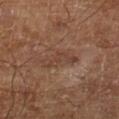Q: Was this lesion biopsied?
A: no biopsy performed (imaged during a skin exam)
Q: Patient demographics?
A: male, in their mid- to late 60s
Q: Illumination type?
A: cross-polarized illumination
Q: How was this image acquired?
A: ~15 mm crop, total-body skin-cancer survey
Q: Lesion size?
A: ~4 mm (longest diameter)